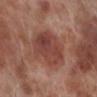biopsy status: catalogued during a skin exam; not biopsied | image: 15 mm crop, total-body photography | body site: the leg | subject: male, about 70 years old.